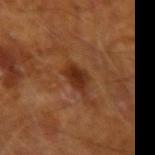The lesion was tiled from a total-body skin photograph and was not biopsied.
A male patient, approximately 65 years of age.
The lesion is located on the left upper arm.
A lesion tile, about 15 mm wide, cut from a 3D total-body photograph.